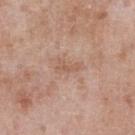{"biopsy_status": "not biopsied; imaged during a skin examination", "lighting": "white-light", "automated_metrics": {"border_irregularity_0_10": 3.5, "peripheral_color_asymmetry": 0.0, "nevus_likeness_0_100": 0}, "lesion_size": {"long_diameter_mm_approx": 2.5}, "site": "chest", "image": {"source": "total-body photography crop", "field_of_view_mm": 15}, "patient": {"sex": "male", "age_approx": 50}}